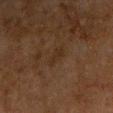biopsy status: catalogued during a skin exam; not biopsied
diameter: ~2.5 mm (longest diameter)
TBP lesion metrics: a footprint of about 3 mm², an outline eccentricity of about 0.85 (0 = round, 1 = elongated), and two-axis asymmetry of about 0.3; a lesion color around L≈22 a*≈13 b*≈22 in CIELAB, about 4 CIELAB-L* units darker than the surrounding skin, and a normalized lesion–skin contrast near 5; a nevus-likeness score of about 0/100 and a detector confidence of about 100 out of 100 that the crop contains a lesion
illumination: cross-polarized
acquisition: 15 mm crop, total-body photography
anatomic site: the chest
subject: male, aged approximately 65